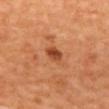This lesion was catalogued during total-body skin photography and was not selected for biopsy. From the mid back. Cropped from a whole-body photographic skin survey; the tile spans about 15 mm. A female patient.